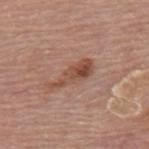No biopsy was performed on this lesion — it was imaged during a full skin examination and was not determined to be concerning.
A male patient roughly 80 years of age.
The lesion's longest dimension is about 5.5 mm.
Imaged with white-light lighting.
Located on the mid back.
This image is a 15 mm lesion crop taken from a total-body photograph.